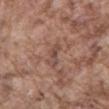workup=catalogued during a skin exam; not biopsied
acquisition=total-body-photography crop, ~15 mm field of view
lesion diameter=~3 mm (longest diameter)
tile lighting=white-light illumination
anatomic site=the front of the torso
patient=male, in their mid- to late 70s
automated metrics=a mean CIELAB color near L≈47 a*≈19 b*≈24, a lesion–skin lightness drop of about 8, and a normalized lesion–skin contrast near 6; border irregularity of about 4 on a 0–10 scale and a peripheral color-asymmetry measure near 0; a lesion-detection confidence of about 95/100